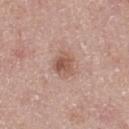biopsy_status: not biopsied; imaged during a skin examination
patient:
  sex: female
  age_approx: 55
lighting: white-light
lesion_size:
  long_diameter_mm_approx: 3.0
site: back
image:
  source: total-body photography crop
  field_of_view_mm: 15